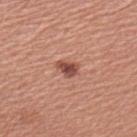Imaged during a routine full-body skin examination; the lesion was not biopsied and no histopathology is available.
Imaged with white-light lighting.
The lesion is located on the left upper arm.
Approximately 2.5 mm at its widest.
This image is a 15 mm lesion crop taken from a total-body photograph.
A female subject aged around 50.